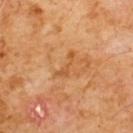follow-up: imaged on a skin check; not biopsied | acquisition: 15 mm crop, total-body photography | patient: male, aged 58–62 | site: the chest.